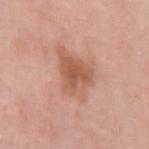Imaged during a routine full-body skin examination; the lesion was not biopsied and no histopathology is available. The tile uses white-light illumination. Approximately 5 mm at its widest. The lesion is on the left upper arm. The patient is a female approximately 45 years of age. A close-up tile cropped from a whole-body skin photograph, about 15 mm across.